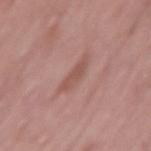Clinical impression:
No biopsy was performed on this lesion — it was imaged during a full skin examination and was not determined to be concerning.
Acquisition and patient details:
Measured at roughly 4 mm in maximum diameter. Located on the lower back. A male subject aged 48–52. Cropped from a whole-body photographic skin survey; the tile spans about 15 mm. Imaged with white-light lighting.